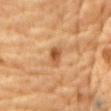Q: Was this lesion biopsied?
A: catalogued during a skin exam; not biopsied
Q: Who is the patient?
A: male, in their mid- to late 80s or older
Q: What is the imaging modality?
A: 15 mm crop, total-body photography
Q: Where on the body is the lesion?
A: the chest